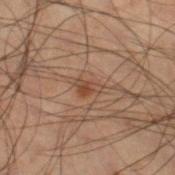workup = imaged on a skin check; not biopsied | lighting = cross-polarized | imaging modality = 15 mm crop, total-body photography | size = ~2.5 mm (longest diameter) | body site = the right lower leg | patient = male, aged around 50.